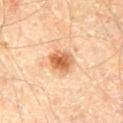Notes:
* biopsy status — no biopsy performed (imaged during a skin exam)
* TBP lesion metrics — a lesion area of about 7.5 mm², an eccentricity of roughly 0.3, and two-axis asymmetry of about 0.25; about 15 CIELAB-L* units darker than the surrounding skin and a normalized border contrast of about 9; a border-irregularity index near 2.5/10, a within-lesion color-variation index near 6/10, and radial color variation of about 2; an automated nevus-likeness rating near 95 out of 100 and lesion-presence confidence of about 100/100
* anatomic site — the mid back
* subject — male, aged 63 to 67
* lesion diameter — about 3 mm
* imaging modality — total-body-photography crop, ~15 mm field of view
* illumination — cross-polarized illumination